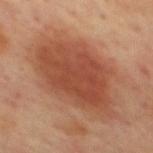follow-up = total-body-photography surveillance lesion; no biopsy | site = the mid back | illumination = cross-polarized illumination | acquisition = 15 mm crop, total-body photography | patient = male, about 65 years old | lesion diameter = about 9.5 mm | TBP lesion metrics = an area of roughly 46 mm², a shape eccentricity near 0.65, and two-axis asymmetry of about 0.25; an average lesion color of about L≈47 a*≈25 b*≈32 (CIELAB), a lesion–skin lightness drop of about 10, and a normalized border contrast of about 8; a border-irregularity index near 3/10, internal color variation of about 3.5 on a 0–10 scale, and peripheral color asymmetry of about 1.